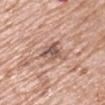• follow-up: no biopsy performed (imaged during a skin exam)
• lesion diameter: about 3 mm
• lighting: white-light
• location: the right upper arm
• subject: male, in their 70s
• image source: ~15 mm crop, total-body skin-cancer survey
• automated lesion analysis: a lesion area of about 6.5 mm², an eccentricity of roughly 0.2, and two-axis asymmetry of about 0.3; a lesion color around L≈55 a*≈20 b*≈25 in CIELAB, a lesion–skin lightness drop of about 11, and a normalized border contrast of about 7.5; a detector confidence of about 100 out of 100 that the crop contains a lesion